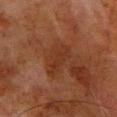Case summary:
- follow-up — no biopsy performed (imaged during a skin exam)
- lesion diameter — about 4.5 mm
- body site — the chest
- patient — male, approximately 80 years of age
- lighting — cross-polarized
- acquisition — ~15 mm crop, total-body skin-cancer survey